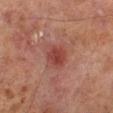Clinical impression:
Part of a total-body skin-imaging series; this lesion was reviewed on a skin check and was not flagged for biopsy.
Acquisition and patient details:
From the left lower leg. Cropped from a total-body skin-imaging series; the visible field is about 15 mm. Imaged with cross-polarized lighting. The subject is a male in their 70s. The lesion's longest dimension is about 2.5 mm.